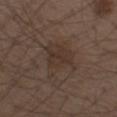notes = no biopsy performed (imaged during a skin exam)